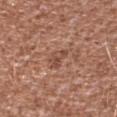<tbp_lesion>
<biopsy_status>not biopsied; imaged during a skin examination</biopsy_status>
<lesion_size>
  <long_diameter_mm_approx>3.0</long_diameter_mm_approx>
</lesion_size>
<site>right upper arm</site>
<patient>
  <sex>male</sex>
  <age_approx>45</age_approx>
</patient>
<image>
  <source>total-body photography crop</source>
  <field_of_view_mm>15</field_of_view_mm>
</image>
<automated_metrics>
  <eccentricity>0.9</eccentricity>
  <cielab_L>48</cielab_L>
  <cielab_a>23</cielab_a>
  <cielab_b>28</cielab_b>
  <vs_skin_darker_L>8.0</vs_skin_darker_L>
  <border_irregularity_0_10>5.0</border_irregularity_0_10>
  <color_variation_0_10>2.5</color_variation_0_10>
  <peripheral_color_asymmetry>0.5</peripheral_color_asymmetry>
  <nevus_likeness_0_100>0</nevus_likeness_0_100>
</automated_metrics>
<lighting>white-light</lighting>
</tbp_lesion>